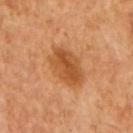<lesion>
  <biopsy_status>not biopsied; imaged during a skin examination</biopsy_status>
  <lighting>cross-polarized</lighting>
  <patient>
    <sex>male</sex>
    <age_approx>65</age_approx>
  </patient>
  <image>
    <source>total-body photography crop</source>
    <field_of_view_mm>15</field_of_view_mm>
  </image>
  <lesion_size>
    <long_diameter_mm_approx>5.0</long_diameter_mm_approx>
  </lesion_size>
  <automated_metrics>
    <area_mm2_approx>14.0</area_mm2_approx>
    <nevus_likeness_0_100>85</nevus_likeness_0_100>
    <lesion_detection_confidence_0_100>100</lesion_detection_confidence_0_100>
  </automated_metrics>
</lesion>